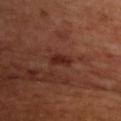Q: Was this lesion biopsied?
A: imaged on a skin check; not biopsied
Q: Automated lesion metrics?
A: a footprint of about 3.5 mm², an outline eccentricity of about 0.85 (0 = round, 1 = elongated), and a symmetry-axis asymmetry near 0.25
Q: What kind of image is this?
A: ~15 mm crop, total-body skin-cancer survey
Q: What are the patient's age and sex?
A: male, aged around 70
Q: What is the anatomic site?
A: the chest